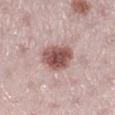Findings:
* follow-up · catalogued during a skin exam; not biopsied
* lesion diameter · about 4 mm
* imaging modality · ~15 mm crop, total-body skin-cancer survey
* lighting · white-light
* subject · female, aged around 25
* body site · the right lower leg